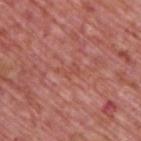Q: Was this lesion biopsied?
A: no biopsy performed (imaged during a skin exam)
Q: Who is the patient?
A: male, about 75 years old
Q: Lesion location?
A: the back
Q: What lighting was used for the tile?
A: white-light
Q: What did automated image analysis measure?
A: a footprint of about 3 mm², a shape eccentricity near 0.9, and two-axis asymmetry of about 0.6; a border-irregularity index near 7.5/10 and a within-lesion color-variation index near 0/10
Q: How was this image acquired?
A: ~15 mm crop, total-body skin-cancer survey
Q: What is the lesion's diameter?
A: about 3 mm